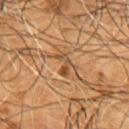workup = imaged on a skin check; not biopsied | anatomic site = the chest | acquisition = ~15 mm crop, total-body skin-cancer survey | patient = male, aged around 55 | tile lighting = cross-polarized.